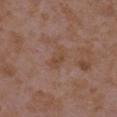The lesion was photographed on a routine skin check and not biopsied; there is no pathology result.